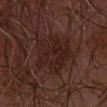Case summary:
• biopsy status · total-body-photography surveillance lesion; no biopsy
• subject · male, in their mid- to late 60s
• image · ~15 mm crop, total-body skin-cancer survey
• location · the left forearm